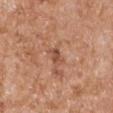Part of a total-body skin-imaging series; this lesion was reviewed on a skin check and was not flagged for biopsy.
From the right upper arm.
A male patient, in their mid-60s.
The tile uses white-light illumination.
An algorithmic analysis of the crop reported a classifier nevus-likeness of about 0/100 and a detector confidence of about 100 out of 100 that the crop contains a lesion.
A 15 mm close-up tile from a total-body photography series done for melanoma screening.
Measured at roughly 3 mm in maximum diameter.